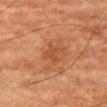{
  "biopsy_status": "not biopsied; imaged during a skin examination",
  "site": "right thigh",
  "patient": {
    "sex": "male",
    "age_approx": 80
  },
  "image": {
    "source": "total-body photography crop",
    "field_of_view_mm": 15
  },
  "lighting": "cross-polarized",
  "lesion_size": {
    "long_diameter_mm_approx": 3.0
  }
}